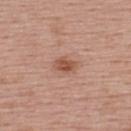Clinical impression: Recorded during total-body skin imaging; not selected for excision or biopsy. Context: On the upper back. A female subject about 55 years old. A 15 mm close-up extracted from a 3D total-body photography capture. The recorded lesion diameter is about 2.5 mm. Automated tile analysis of the lesion measured an eccentricity of roughly 0.7 and a symmetry-axis asymmetry near 0.15. And it measured a border-irregularity rating of about 1.5/10 and internal color variation of about 4 on a 0–10 scale. Imaged with white-light lighting.